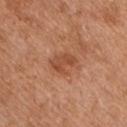biopsy_status: not biopsied; imaged during a skin examination
automated_metrics:
  area_mm2_approx: 6.0
  eccentricity: 0.75
  shape_asymmetry: 0.35
  cielab_L: 49
  cielab_a: 26
  cielab_b: 34
  vs_skin_darker_L: 9.0
  border_irregularity_0_10: 4.0
  peripheral_color_asymmetry: 0.5
  nevus_likeness_0_100: 35
lighting: white-light
image:
  source: total-body photography crop
  field_of_view_mm: 15
site: chest
patient:
  sex: male
  age_approx: 55